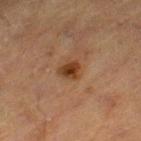Clinical impression:
The lesion was photographed on a routine skin check and not biopsied; there is no pathology result.
Context:
The patient is a male in their mid-80s or older. Cropped from a total-body skin-imaging series; the visible field is about 15 mm. From the leg.